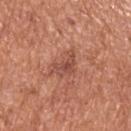Q: Was a biopsy performed?
A: no biopsy performed (imaged during a skin exam)
Q: Automated lesion metrics?
A: a shape eccentricity near 0.8 and two-axis asymmetry of about 0.5; a border-irregularity index near 5.5/10, internal color variation of about 3 on a 0–10 scale, and peripheral color asymmetry of about 0.5
Q: What are the patient's age and sex?
A: male, roughly 65 years of age
Q: How was this image acquired?
A: total-body-photography crop, ~15 mm field of view
Q: Lesion location?
A: the back